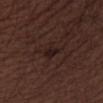This lesion was catalogued during total-body skin photography and was not selected for biopsy.
A male patient, about 50 years old.
The lesion is on the right thigh.
A region of skin cropped from a whole-body photographic capture, roughly 15 mm wide.